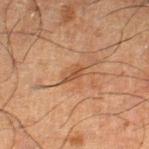Impression:
Part of a total-body skin-imaging series; this lesion was reviewed on a skin check and was not flagged for biopsy.
Context:
The recorded lesion diameter is about 2.5 mm. The lesion is located on the right lower leg. A male patient aged 63 to 67. An algorithmic analysis of the crop reported a footprint of about 2.5 mm², an eccentricity of roughly 0.9, and a symmetry-axis asymmetry near 0.35. And it measured a mean CIELAB color near L≈41 a*≈19 b*≈30 and a normalized lesion–skin contrast near 6.5. It also reported a border-irregularity index near 3.5/10 and a color-variation rating of about 0/10. And it measured a nevus-likeness score of about 0/100 and lesion-presence confidence of about 95/100. Cropped from a total-body skin-imaging series; the visible field is about 15 mm. The tile uses cross-polarized illumination.